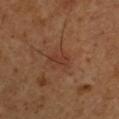Q: Was this lesion biopsied?
A: total-body-photography surveillance lesion; no biopsy
Q: What are the patient's age and sex?
A: male, aged 48–52
Q: What is the imaging modality?
A: ~15 mm crop, total-body skin-cancer survey
Q: Where on the body is the lesion?
A: the chest